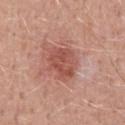{"biopsy_status": "not biopsied; imaged during a skin examination", "patient": {"sex": "male", "age_approx": 50}, "lesion_size": {"long_diameter_mm_approx": 4.0}, "image": {"source": "total-body photography crop", "field_of_view_mm": 15}, "automated_metrics": {"eccentricity": 0.55, "shape_asymmetry": 0.25, "nevus_likeness_0_100": 10, "lesion_detection_confidence_0_100": 100}, "site": "abdomen"}